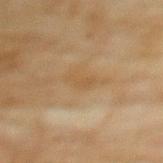Impression:
Recorded during total-body skin imaging; not selected for excision or biopsy.
Clinical summary:
An algorithmic analysis of the crop reported a footprint of about 3 mm², a shape eccentricity near 0.9, and two-axis asymmetry of about 0.4. The analysis additionally found a mean CIELAB color near L≈46 a*≈14 b*≈33, roughly 5 lightness units darker than nearby skin, and a normalized border contrast of about 5. The analysis additionally found a nevus-likeness score of about 0/100 and a detector confidence of about 100 out of 100 that the crop contains a lesion. A female subject in their 80s. From the back. A region of skin cropped from a whole-body photographic capture, roughly 15 mm wide.